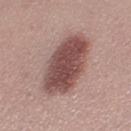Q: Is there a histopathology result?
A: catalogued during a skin exam; not biopsied
Q: How was this image acquired?
A: ~15 mm crop, total-body skin-cancer survey
Q: Patient demographics?
A: female, in their mid-20s
Q: Lesion location?
A: the right thigh